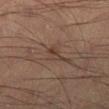  biopsy_status: not biopsied; imaged during a skin examination
  automated_metrics:
    area_mm2_approx: 3.0
    eccentricity: 0.85
    cielab_L: 34
    cielab_a: 15
    cielab_b: 22
    vs_skin_darker_L: 6.0
    vs_skin_contrast_norm: 6.0
    border_irregularity_0_10: 3.0
    color_variation_0_10: 4.0
    peripheral_color_asymmetry: 1.5
    nevus_likeness_0_100: 0
    lesion_detection_confidence_0_100: 65
  site: right lower leg
  image:
    source: total-body photography crop
    field_of_view_mm: 15
  lesion_size:
    long_diameter_mm_approx: 2.5
  lighting: cross-polarized
  patient:
    sex: male
    age_approx: 40